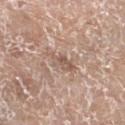body site: the left lower leg
illumination: white-light
image source: total-body-photography crop, ~15 mm field of view
automated metrics: a lesion color around L≈57 a*≈17 b*≈26 in CIELAB, roughly 9 lightness units darker than nearby skin, and a lesion-to-skin contrast of about 6 (normalized; higher = more distinct); a classifier nevus-likeness of about 0/100 and a detector confidence of about 60 out of 100 that the crop contains a lesion
patient: female, in their mid- to late 70s